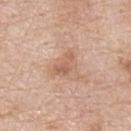Clinical impression:
No biopsy was performed on this lesion — it was imaged during a full skin examination and was not determined to be concerning.
Image and clinical context:
The subject is a male aged around 80. Captured under white-light illumination. A lesion tile, about 15 mm wide, cut from a 3D total-body photograph. Located on the upper back.